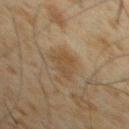Q: Lesion size?
A: about 3.5 mm
Q: Patient demographics?
A: male, in their 60s
Q: Illumination type?
A: cross-polarized
Q: Where on the body is the lesion?
A: the front of the torso
Q: How was this image acquired?
A: 15 mm crop, total-body photography
Q: Automated lesion metrics?
A: a footprint of about 5.5 mm², an outline eccentricity of about 0.75 (0 = round, 1 = elongated), and two-axis asymmetry of about 0.35; a mean CIELAB color near L≈39 a*≈12 b*≈28, a lesion–skin lightness drop of about 5, and a normalized lesion–skin contrast near 6; a border-irregularity rating of about 4/10, internal color variation of about 2.5 on a 0–10 scale, and peripheral color asymmetry of about 1; a classifier nevus-likeness of about 0/100